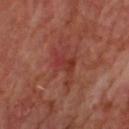Assessment:
No biopsy was performed on this lesion — it was imaged during a full skin examination and was not determined to be concerning.
Context:
The lesion's longest dimension is about 5 mm. The lesion is on the back. Automated tile analysis of the lesion measured an area of roughly 8.5 mm² and a symmetry-axis asymmetry near 0.5. The software also gave a lesion color around L≈36 a*≈28 b*≈26 in CIELAB. The analysis additionally found border irregularity of about 7.5 on a 0–10 scale, a within-lesion color-variation index near 4/10, and a peripheral color-asymmetry measure near 1. The software also gave a detector confidence of about 90 out of 100 that the crop contains a lesion. A region of skin cropped from a whole-body photographic capture, roughly 15 mm wide. The tile uses cross-polarized illumination. A male subject roughly 65 years of age.